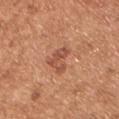Assessment:
No biopsy was performed on this lesion — it was imaged during a full skin examination and was not determined to be concerning.
Context:
The tile uses white-light illumination. On the chest. A male subject, in their mid- to late 50s. A region of skin cropped from a whole-body photographic capture, roughly 15 mm wide. The recorded lesion diameter is about 3 mm.